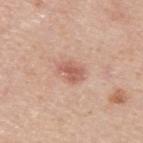Clinical impression: Part of a total-body skin-imaging series; this lesion was reviewed on a skin check and was not flagged for biopsy. Context: The subject is a male roughly 45 years of age. The lesion is located on the back. Captured under white-light illumination. A lesion tile, about 15 mm wide, cut from a 3D total-body photograph.